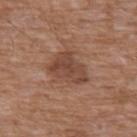{
  "biopsy_status": "not biopsied; imaged during a skin examination",
  "automated_metrics": {
    "nevus_likeness_0_100": 5
  },
  "site": "chest",
  "patient": {
    "sex": "male",
    "age_approx": 60
  },
  "lighting": "white-light",
  "image": {
    "source": "total-body photography crop",
    "field_of_view_mm": 15
  },
  "lesion_size": {
    "long_diameter_mm_approx": 5.0
  }
}